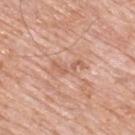Assessment: The lesion was photographed on a routine skin check and not biopsied; there is no pathology result. Acquisition and patient details: The patient is a male about 80 years old. The tile uses white-light illumination. From the upper back. A lesion tile, about 15 mm wide, cut from a 3D total-body photograph. About 4 mm across.